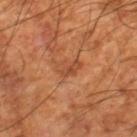biopsy status: total-body-photography surveillance lesion; no biopsy
patient: male, aged approximately 60
imaging modality: ~15 mm tile from a whole-body skin photo
size: about 3 mm
lighting: cross-polarized
anatomic site: the leg